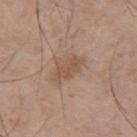Impression: The lesion was tiled from a total-body skin photograph and was not biopsied. Background: Located on the upper back. Cropped from a total-body skin-imaging series; the visible field is about 15 mm. A male patient aged around 55. The recorded lesion diameter is about 4 mm. Captured under white-light illumination. An algorithmic analysis of the crop reported a shape eccentricity near 0.9 and two-axis asymmetry of about 0.3. And it measured a lesion color around L≈52 a*≈18 b*≈29 in CIELAB, a lesion–skin lightness drop of about 8, and a normalized border contrast of about 6. It also reported a color-variation rating of about 2/10. The software also gave a classifier nevus-likeness of about 5/100 and a detector confidence of about 100 out of 100 that the crop contains a lesion.